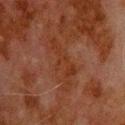Q: Was this lesion biopsied?
A: catalogued during a skin exam; not biopsied
Q: What lighting was used for the tile?
A: cross-polarized
Q: Lesion size?
A: about 6.5 mm
Q: How was this image acquired?
A: ~15 mm crop, total-body skin-cancer survey
Q: Patient demographics?
A: male, aged approximately 80
Q: What is the anatomic site?
A: the back